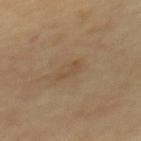Findings:
– notes · catalogued during a skin exam; not biopsied
– lesion size · ~2.5 mm (longest diameter)
– acquisition · ~15 mm tile from a whole-body skin photo
– illumination · cross-polarized
– body site · the back
– patient · female, in their 60s